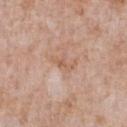Part of a total-body skin-imaging series; this lesion was reviewed on a skin check and was not flagged for biopsy.
A lesion tile, about 15 mm wide, cut from a 3D total-body photograph.
This is a white-light tile.
The lesion is located on the front of the torso.
The recorded lesion diameter is about 3 mm.
A male subject, aged 58 to 62.